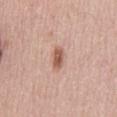<record>
<biopsy_status>not biopsied; imaged during a skin examination</biopsy_status>
<image>
  <source>total-body photography crop</source>
  <field_of_view_mm>15</field_of_view_mm>
</image>
<lesion_size>
  <long_diameter_mm_approx>3.0</long_diameter_mm_approx>
</lesion_size>
<site>front of the torso</site>
<patient>
  <sex>male</sex>
  <age_approx>65</age_approx>
</patient>
<automated_metrics>
  <area_mm2_approx>4.5</area_mm2_approx>
  <eccentricity>0.9</eccentricity>
  <cielab_L>57</cielab_L>
  <cielab_a>22</cielab_a>
  <cielab_b>29</cielab_b>
  <vs_skin_darker_L>13.0</vs_skin_darker_L>
  <vs_skin_contrast_norm>8.5</vs_skin_contrast_norm>
</automated_metrics>
</record>